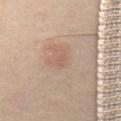Impression:
This lesion was catalogued during total-body skin photography and was not selected for biopsy.
Context:
Automated image analysis of the tile measured border irregularity of about 3 on a 0–10 scale and a peripheral color-asymmetry measure near 0. The software also gave a nevus-likeness score of about 0/100 and lesion-presence confidence of about 100/100. Approximately 1.5 mm at its widest. The lesion is located on the abdomen. Imaged with cross-polarized lighting. A close-up tile cropped from a whole-body skin photograph, about 15 mm across. A female subject aged 43–47.